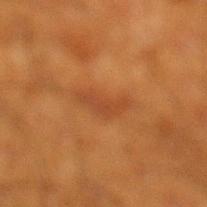biopsy status — total-body-photography surveillance lesion; no biopsy | diameter — about 5 mm | subject — male, in their 60s | lighting — cross-polarized | TBP lesion metrics — a border-irregularity index near 5.5/10, a within-lesion color-variation index near 2.5/10, and radial color variation of about 1 | image — ~15 mm tile from a whole-body skin photo | body site — the leg.